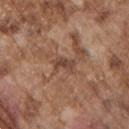Assessment:
Captured during whole-body skin photography for melanoma surveillance; the lesion was not biopsied.
Context:
A male subject aged around 75. Captured under white-light illumination. The lesion is on the chest. A region of skin cropped from a whole-body photographic capture, roughly 15 mm wide.